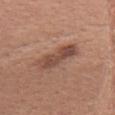{
  "biopsy_status": "not biopsied; imaged during a skin examination",
  "patient": {
    "sex": "female",
    "age_approx": 50
  },
  "site": "head or neck",
  "image": {
    "source": "total-body photography crop",
    "field_of_view_mm": 15
  }
}